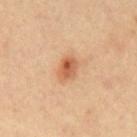The lesion was tiled from a total-body skin photograph and was not biopsied. A female subject in their mid-40s. Measured at roughly 3 mm in maximum diameter. Automated image analysis of the tile measured border irregularity of about 1.5 on a 0–10 scale, a color-variation rating of about 7/10, and peripheral color asymmetry of about 2. The analysis additionally found a nevus-likeness score of about 90/100. Located on the mid back. Imaged with cross-polarized lighting. A region of skin cropped from a whole-body photographic capture, roughly 15 mm wide.